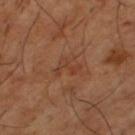Case summary:
* biopsy status — total-body-photography surveillance lesion; no biopsy
* anatomic site — the left thigh
* image source — total-body-photography crop, ~15 mm field of view
* subject — male, about 65 years old
* diameter — ≈3 mm
* lighting — cross-polarized illumination